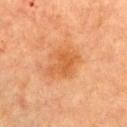Case summary:
* biopsy status · catalogued during a skin exam; not biopsied
* subject · female, in their 60s
* body site · the chest
* image · total-body-photography crop, ~15 mm field of view
* lesion diameter · ≈4.5 mm